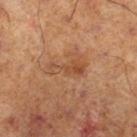| field | value |
|---|---|
| follow-up | imaged on a skin check; not biopsied |
| lesion diameter | ≈4 mm |
| anatomic site | the right lower leg |
| imaging modality | ~15 mm tile from a whole-body skin photo |
| patient | male, aged approximately 70 |